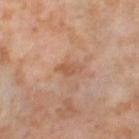No biopsy was performed on this lesion — it was imaged during a full skin examination and was not determined to be concerning.
The lesion is on the leg.
A roughly 15 mm field-of-view crop from a total-body skin photograph.
The patient is a female aged around 55.